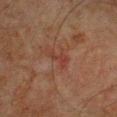notes: imaged on a skin check; not biopsied | imaging modality: ~15 mm crop, total-body skin-cancer survey | lesion diameter: ~3 mm (longest diameter) | site: the chest | patient: male, aged 58 to 62 | lighting: cross-polarized.